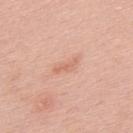follow-up = imaged on a skin check; not biopsied | acquisition = 15 mm crop, total-body photography | lesion diameter = about 3 mm | anatomic site = the upper back | lighting = white-light | subject = male, aged approximately 55.